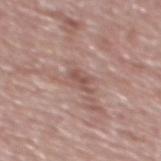<lesion>
  <biopsy_status>not biopsied; imaged during a skin examination</biopsy_status>
  <image>
    <source>total-body photography crop</source>
    <field_of_view_mm>15</field_of_view_mm>
  </image>
  <automated_metrics>
    <eccentricity>0.85</eccentricity>
    <cielab_L>51</cielab_L>
    <cielab_a>20</cielab_a>
    <cielab_b>23</cielab_b>
    <vs_skin_darker_L>9.0</vs_skin_darker_L>
    <border_irregularity_0_10>4.5</border_irregularity_0_10>
    <color_variation_0_10>0.0</color_variation_0_10>
    <peripheral_color_asymmetry>0.0</peripheral_color_asymmetry>
  </automated_metrics>
  <lighting>white-light</lighting>
  <patient>
    <sex>male</sex>
    <age_approx>70</age_approx>
  </patient>
  <site>mid back</site>
  <lesion_size>
    <long_diameter_mm_approx>2.5</long_diameter_mm_approx>
  </lesion_size>
</lesion>